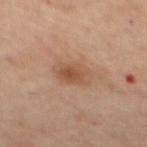Recorded during total-body skin imaging; not selected for excision or biopsy. Measured at roughly 3.5 mm in maximum diameter. Captured under cross-polarized illumination. A female patient in their mid- to late 40s. On the mid back. Cropped from a total-body skin-imaging series; the visible field is about 15 mm. The total-body-photography lesion software estimated a border-irregularity index near 2/10, a color-variation rating of about 4/10, and peripheral color asymmetry of about 1.5. The software also gave a nevus-likeness score of about 50/100 and lesion-presence confidence of about 100/100.